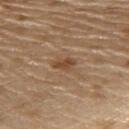Findings:
- notes: imaged on a skin check; not biopsied
- patient: male, aged around 70
- image: ~15 mm tile from a whole-body skin photo
- site: the upper back